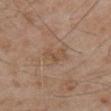The lesion was tiled from a total-body skin photograph and was not biopsied. The tile uses white-light illumination. A 15 mm close-up extracted from a 3D total-body photography capture. A male patient about 55 years old. The lesion is located on the arm. Approximately 2.5 mm at its widest.